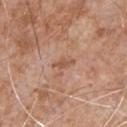Q: Was this lesion biopsied?
A: total-body-photography surveillance lesion; no biopsy
Q: What are the patient's age and sex?
A: male, aged approximately 65
Q: What kind of image is this?
A: total-body-photography crop, ~15 mm field of view
Q: Where on the body is the lesion?
A: the front of the torso
Q: How large is the lesion?
A: ~2.5 mm (longest diameter)